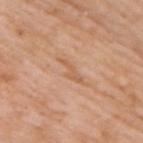Clinical impression:
This lesion was catalogued during total-body skin photography and was not selected for biopsy.
Clinical summary:
A male patient approximately 60 years of age. On the right upper arm. A 15 mm close-up tile from a total-body photography series done for melanoma screening.